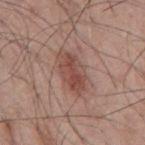Recorded during total-body skin imaging; not selected for excision or biopsy. The subject is a male about 55 years old. A close-up tile cropped from a whole-body skin photograph, about 15 mm across. Automated tile analysis of the lesion measured a lesion area of about 12 mm² and an outline eccentricity of about 0.85 (0 = round, 1 = elongated). On the back. The recorded lesion diameter is about 5.5 mm. This is a white-light tile.